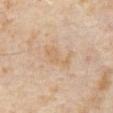Captured during whole-body skin photography for melanoma surveillance; the lesion was not biopsied.
A roughly 15 mm field-of-view crop from a total-body skin photograph.
Approximately 4.5 mm at its widest.
The tile uses cross-polarized illumination.
The subject is a male aged around 65.